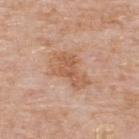{"patient": {"sex": "male", "age_approx": 75}, "image": {"source": "total-body photography crop", "field_of_view_mm": 15}, "site": "upper back", "lighting": "white-light"}